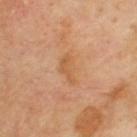notes — total-body-photography surveillance lesion; no biopsy
body site — the upper back
acquisition — total-body-photography crop, ~15 mm field of view
subject — male, approximately 70 years of age
lighting — cross-polarized illumination
lesion size — about 4 mm
automated metrics — an automated nevus-likeness rating near 0 out of 100 and lesion-presence confidence of about 100/100